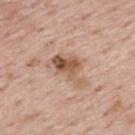Imaged with white-light lighting.
Measured at roughly 5 mm in maximum diameter.
The lesion-visualizer software estimated an area of roughly 9 mm², an eccentricity of roughly 0.8, and a shape-asymmetry score of about 0.5 (0 = symmetric). And it measured a border-irregularity rating of about 6/10 and internal color variation of about 7 on a 0–10 scale.
A roughly 15 mm field-of-view crop from a total-body skin photograph.
The lesion is on the mid back.
The patient is a male aged approximately 75.